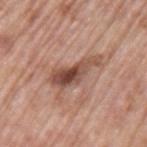This lesion was catalogued during total-body skin photography and was not selected for biopsy. Measured at roughly 6 mm in maximum diameter. A close-up tile cropped from a whole-body skin photograph, about 15 mm across. This is a white-light tile. An algorithmic analysis of the crop reported an eccentricity of roughly 0.95 and a symmetry-axis asymmetry near 0.4. The analysis additionally found an average lesion color of about L≈49 a*≈22 b*≈27 (CIELAB), roughly 13 lightness units darker than nearby skin, and a normalized border contrast of about 9.5. The analysis additionally found a lesion-detection confidence of about 100/100. A male patient aged 68 to 72. The lesion is on the left upper arm.